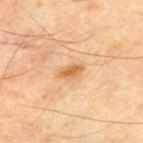Recorded during total-body skin imaging; not selected for excision or biopsy.
Approximately 3 mm at its widest.
The patient is a male in their 70s.
A lesion tile, about 15 mm wide, cut from a 3D total-body photograph.
Located on the front of the torso.
Imaged with cross-polarized lighting.